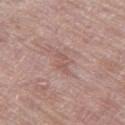notes: imaged on a skin check; not biopsied | lesion diameter: ~3 mm (longest diameter) | body site: the leg | patient: male, aged 63–67 | image source: ~15 mm crop, total-body skin-cancer survey | automated metrics: roughly 7 lightness units darker than nearby skin and a lesion-to-skin contrast of about 5 (normalized; higher = more distinct); a border-irregularity rating of about 5.5/10, a color-variation rating of about 0/10, and radial color variation of about 0; a nevus-likeness score of about 0/100 and lesion-presence confidence of about 90/100 | illumination: white-light.